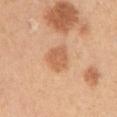workup=imaged on a skin check; not biopsied | tile lighting=white-light | lesion size=≈3 mm | TBP lesion metrics=a lesion color around L≈62 a*≈24 b*≈37 in CIELAB, a lesion–skin lightness drop of about 10, and a normalized lesion–skin contrast near 6.5; a border-irregularity rating of about 2/10, a color-variation rating of about 2/10, and a peripheral color-asymmetry measure near 0.5; an automated nevus-likeness rating near 70 out of 100 and a lesion-detection confidence of about 100/100 | body site=the left upper arm | acquisition=~15 mm tile from a whole-body skin photo | patient=female, aged approximately 45.